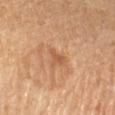Assessment: The lesion was tiled from a total-body skin photograph and was not biopsied.